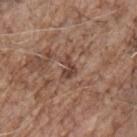A 15 mm close-up tile from a total-body photography series done for melanoma screening.
The tile uses white-light illumination.
Longest diameter approximately 3 mm.
On the chest.
A male subject, aged 68–72.